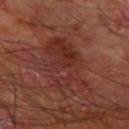Case summary:
* automated metrics · a footprint of about 22 mm², a shape eccentricity near 0.8, and a shape-asymmetry score of about 0.25 (0 = symmetric); a mean CIELAB color near L≈32 a*≈25 b*≈24 and a lesion-to-skin contrast of about 6 (normalized; higher = more distinct); a classifier nevus-likeness of about 0/100 and lesion-presence confidence of about 95/100
* image source · ~15 mm tile from a whole-body skin photo
* subject · male, in their mid-60s
* lesion size · ≈7 mm
* tile lighting · cross-polarized illumination
* location · the right forearm